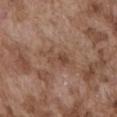Assessment: No biopsy was performed on this lesion — it was imaged during a full skin examination and was not determined to be concerning. Acquisition and patient details: A close-up tile cropped from a whole-body skin photograph, about 15 mm across. About 5 mm across. This is a white-light tile. From the abdomen. A male subject aged approximately 75.